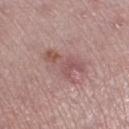No biopsy was performed on this lesion — it was imaged during a full skin examination and was not determined to be concerning.
The lesion is located on the left lower leg.
A close-up tile cropped from a whole-body skin photograph, about 15 mm across.
A female subject roughly 45 years of age.